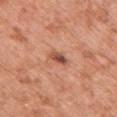The lesion was tiled from a total-body skin photograph and was not biopsied. The total-body-photography lesion software estimated a shape eccentricity near 0.9 and a symmetry-axis asymmetry near 0.2. It also reported a mean CIELAB color near L≈51 a*≈26 b*≈31, about 14 CIELAB-L* units darker than the surrounding skin, and a lesion-to-skin contrast of about 9.5 (normalized; higher = more distinct). The software also gave border irregularity of about 2 on a 0–10 scale, a within-lesion color-variation index near 4/10, and a peripheral color-asymmetry measure near 0.5. It also reported an automated nevus-likeness rating near 50 out of 100 and lesion-presence confidence of about 100/100. This image is a 15 mm lesion crop taken from a total-body photograph. Imaged with white-light lighting. A female patient aged 48 to 52. On the front of the torso.